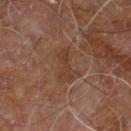Captured during whole-body skin photography for melanoma surveillance; the lesion was not biopsied.
The total-body-photography lesion software estimated a footprint of about 5 mm², an outline eccentricity of about 0.95 (0 = round, 1 = elongated), and two-axis asymmetry of about 0.25. It also reported about 6 CIELAB-L* units darker than the surrounding skin. And it measured a border-irregularity rating of about 4.5/10 and radial color variation of about 0.5.
Captured under cross-polarized illumination.
On the right leg.
A male subject about 60 years old.
A region of skin cropped from a whole-body photographic capture, roughly 15 mm wide.